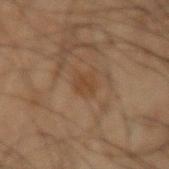Assessment: No biopsy was performed on this lesion — it was imaged during a full skin examination and was not determined to be concerning. Background: Approximately 3 mm at its widest. A roughly 15 mm field-of-view crop from a total-body skin photograph. Located on the left upper arm. A male subject aged approximately 50.